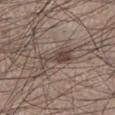{
  "patient": {
    "sex": "male",
    "age_approx": 35
  },
  "site": "left lower leg",
  "image": {
    "source": "total-body photography crop",
    "field_of_view_mm": 15
  }
}